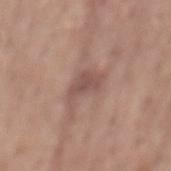Recorded during total-body skin imaging; not selected for excision or biopsy.